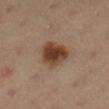Imaged with cross-polarized lighting.
The total-body-photography lesion software estimated an automated nevus-likeness rating near 100 out of 100 and lesion-presence confidence of about 100/100.
A female patient, aged 33–37.
A roughly 15 mm field-of-view crop from a total-body skin photograph.
Measured at roughly 4 mm in maximum diameter.
From the right lower leg.